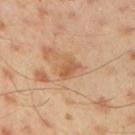No biopsy was performed on this lesion — it was imaged during a full skin examination and was not determined to be concerning.
The total-body-photography lesion software estimated an average lesion color of about L≈57 a*≈21 b*≈36 (CIELAB) and about 8 CIELAB-L* units darker than the surrounding skin. The software also gave border irregularity of about 2 on a 0–10 scale, internal color variation of about 4.5 on a 0–10 scale, and peripheral color asymmetry of about 1.5. The analysis additionally found an automated nevus-likeness rating near 0 out of 100 and lesion-presence confidence of about 100/100.
This is a cross-polarized tile.
A 15 mm close-up extracted from a 3D total-body photography capture.
A male subject in their mid- to late 40s.
The lesion is on the right upper arm.